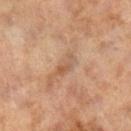Image and clinical context: The tile uses cross-polarized illumination. Longest diameter approximately 3 mm. A female subject in their 60s. From the right lower leg. A 15 mm crop from a total-body photograph taken for skin-cancer surveillance.